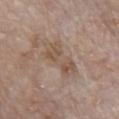diameter: about 5.5 mm
illumination: white-light
patient: male, aged around 85 or older
acquisition: ~15 mm crop, total-body skin-cancer survey
automated metrics: a lesion area of about 11 mm², an outline eccentricity of about 0.9 (0 = round, 1 = elongated), and a shape-asymmetry score of about 0.3 (0 = symmetric); internal color variation of about 4 on a 0–10 scale and peripheral color asymmetry of about 1.5
anatomic site: the chest